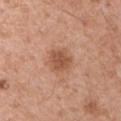Impression:
Recorded during total-body skin imaging; not selected for excision or biopsy.
Background:
On the left upper arm. The patient is a male aged around 55. A region of skin cropped from a whole-body photographic capture, roughly 15 mm wide.